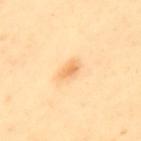Acquisition and patient details: The subject is a female approximately 60 years of age. The lesion is on the upper back. The recorded lesion diameter is about 2.5 mm. A region of skin cropped from a whole-body photographic capture, roughly 15 mm wide.